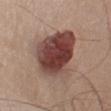The lesion was tiled from a total-body skin photograph and was not biopsied.
Captured under white-light illumination.
A male subject, aged approximately 50.
Cropped from a whole-body photographic skin survey; the tile spans about 15 mm.
From the left upper arm.